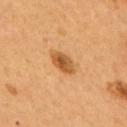workup — total-body-photography surveillance lesion; no biopsy
image — 15 mm crop, total-body photography
location — the upper back
illumination — cross-polarized illumination
patient — male, aged around 55
lesion diameter — ≈3.5 mm